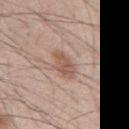biopsy status=catalogued during a skin exam; not biopsied | patient=male, in their mid- to late 40s | location=the mid back | acquisition=~15 mm crop, total-body skin-cancer survey.